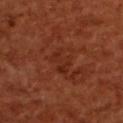Recorded during total-body skin imaging; not selected for excision or biopsy.
On the upper back.
The patient is a female roughly 55 years of age.
The recorded lesion diameter is about 3 mm.
Imaged with cross-polarized lighting.
A roughly 15 mm field-of-view crop from a total-body skin photograph.